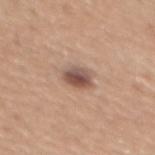follow-up=total-body-photography surveillance lesion; no biopsy
lesion diameter=~3 mm (longest diameter)
subject=female, aged around 55
image source=~15 mm tile from a whole-body skin photo
body site=the mid back